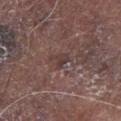Imaged during a routine full-body skin examination; the lesion was not biopsied and no histopathology is available.
A close-up tile cropped from a whole-body skin photograph, about 15 mm across.
On the chest.
Longest diameter approximately 2.5 mm.
A male patient about 80 years old.
This is a white-light tile.
An algorithmic analysis of the crop reported a lesion color around L≈37 a*≈17 b*≈18 in CIELAB, roughly 7 lightness units darker than nearby skin, and a normalized lesion–skin contrast near 6.5.